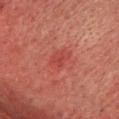follow-up — total-body-photography surveillance lesion; no biopsy
patient — male, approximately 75 years of age
acquisition — total-body-photography crop, ~15 mm field of view
illumination — cross-polarized illumination
location — the head or neck
size — ~3 mm (longest diameter)